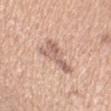Impression: Recorded during total-body skin imaging; not selected for excision or biopsy. Context: The lesion is located on the left upper arm. A female patient aged approximately 50. A lesion tile, about 15 mm wide, cut from a 3D total-body photograph.